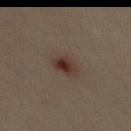biopsy status = total-body-photography surveillance lesion; no biopsy | diameter = ≈2.5 mm | lighting = cross-polarized | image source = ~15 mm crop, total-body skin-cancer survey | patient = male, aged approximately 35 | TBP lesion metrics = a footprint of about 5 mm², a shape eccentricity near 0.65, and a symmetry-axis asymmetry near 0.2; a mean CIELAB color near L≈31 a*≈16 b*≈21 and a normalized lesion–skin contrast near 9.5 | body site = the mid back.